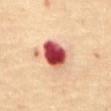The lesion is located on the abdomen. Approximately 4 mm at its widest. An algorithmic analysis of the crop reported an area of roughly 11 mm², an outline eccentricity of about 0.65 (0 = round, 1 = elongated), and a symmetry-axis asymmetry near 0.15. And it measured a lesion color around L≈52 a*≈35 b*≈29 in CIELAB and roughly 25 lightness units darker than nearby skin. And it measured a nevus-likeness score of about 0/100 and lesion-presence confidence of about 100/100. A lesion tile, about 15 mm wide, cut from a 3D total-body photograph. This is a cross-polarized tile. A male subject, approximately 70 years of age.